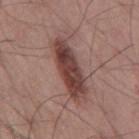biopsy status — total-body-photography surveillance lesion; no biopsy
patient — male, aged around 55
acquisition — 15 mm crop, total-body photography
anatomic site — the mid back